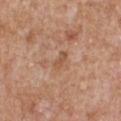  biopsy_status: not biopsied; imaged during a skin examination
  site: chest
  image:
    source: total-body photography crop
    field_of_view_mm: 15
  patient:
    sex: male
    age_approx: 65
  automated_metrics:
    eccentricity: 0.85
    cielab_L: 54
    cielab_a: 21
    cielab_b: 33
    vs_skin_darker_L: 7.0
    vs_skin_contrast_norm: 6.0
    border_irregularity_0_10: 3.0
    color_variation_0_10: 1.0
    peripheral_color_asymmetry: 0.5
  lesion_size:
    long_diameter_mm_approx: 2.5
  lighting: white-light